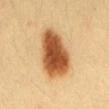biopsy_status: not biopsied; imaged during a skin examination
image:
  source: total-body photography crop
  field_of_view_mm: 15
automated_metrics:
  area_mm2_approx: 23.0
  eccentricity: 0.8
  nevus_likeness_0_100: 100
  lesion_detection_confidence_0_100: 100
lesion_size:
  long_diameter_mm_approx: 7.0
site: mid back
patient:
  sex: female
  age_approx: 30